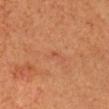Clinical impression:
Captured during whole-body skin photography for melanoma surveillance; the lesion was not biopsied.
Acquisition and patient details:
The lesion is on the head or neck. A close-up tile cropped from a whole-body skin photograph, about 15 mm across. A female subject aged approximately 45.